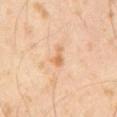Impression: Imaged during a routine full-body skin examination; the lesion was not biopsied and no histopathology is available. Clinical summary: The patient is a male approximately 65 years of age. Longest diameter approximately 2.5 mm. A 15 mm close-up extracted from a 3D total-body photography capture. Captured under cross-polarized illumination. Automated image analysis of the tile measured a lesion color around L≈66 a*≈21 b*≈38 in CIELAB, roughly 9 lightness units darker than nearby skin, and a normalized border contrast of about 7. The software also gave border irregularity of about 5 on a 0–10 scale and internal color variation of about 1 on a 0–10 scale.